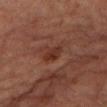Part of a total-body skin-imaging series; this lesion was reviewed on a skin check and was not flagged for biopsy. The tile uses cross-polarized illumination. A close-up tile cropped from a whole-body skin photograph, about 15 mm across. A male patient in their mid-80s or older. The lesion is on the left lower leg.